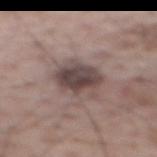<lesion>
<biopsy_status>not biopsied; imaged during a skin examination</biopsy_status>
<automated_metrics>
  <vs_skin_darker_L>13.0</vs_skin_darker_L>
  <vs_skin_contrast_norm>10.0</vs_skin_contrast_norm>
  <border_irregularity_0_10>3.0</border_irregularity_0_10>
  <nevus_likeness_0_100>10</nevus_likeness_0_100>
  <lesion_detection_confidence_0_100>100</lesion_detection_confidence_0_100>
</automated_metrics>
<patient>
  <sex>male</sex>
  <age_approx>70</age_approx>
</patient>
<lesion_size>
  <long_diameter_mm_approx>4.5</long_diameter_mm_approx>
</lesion_size>
<site>upper back</site>
<image>
  <source>total-body photography crop</source>
  <field_of_view_mm>15</field_of_view_mm>
</image>
</lesion>